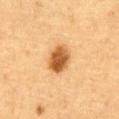Imaged with cross-polarized lighting.
The lesion is on the front of the torso.
About 3.5 mm across.
A male patient aged 83 or over.
A 15 mm close-up extracted from a 3D total-body photography capture.
An algorithmic analysis of the crop reported a lesion area of about 8 mm², an eccentricity of roughly 0.65, and a symmetry-axis asymmetry near 0.15. The analysis additionally found a lesion color around L≈50 a*≈22 b*≈41 in CIELAB, a lesion–skin lightness drop of about 16, and a lesion-to-skin contrast of about 11 (normalized; higher = more distinct). And it measured a border-irregularity index near 1.5/10, a color-variation rating of about 4.5/10, and a peripheral color-asymmetry measure near 1.5. It also reported an automated nevus-likeness rating near 100 out of 100 and a lesion-detection confidence of about 100/100.